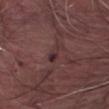workup = total-body-photography surveillance lesion; no biopsy | size = ~2.5 mm (longest diameter) | subject = male, aged 78–82 | site = the left thigh | imaging modality = ~15 mm crop, total-body skin-cancer survey | lighting = white-light illumination.